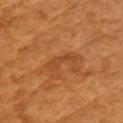Q: Was a biopsy performed?
A: total-body-photography surveillance lesion; no biopsy
Q: Lesion location?
A: the right upper arm
Q: Automated lesion metrics?
A: an outline eccentricity of about 0.9 (0 = round, 1 = elongated); a border-irregularity index near 6/10, a color-variation rating of about 0.5/10, and a peripheral color-asymmetry measure near 0
Q: Illumination type?
A: cross-polarized illumination
Q: What are the patient's age and sex?
A: female, roughly 55 years of age
Q: How large is the lesion?
A: about 3 mm
Q: What is the imaging modality?
A: 15 mm crop, total-body photography